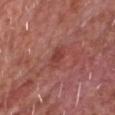No biopsy was performed on this lesion — it was imaged during a full skin examination and was not determined to be concerning. A 15 mm crop from a total-body photograph taken for skin-cancer surveillance. The tile uses white-light illumination. From the head or neck. The subject is a male about 70 years old. Measured at roughly 3 mm in maximum diameter.